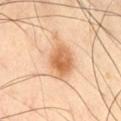A male subject aged approximately 55. A roughly 15 mm field-of-view crop from a total-body skin photograph. Located on the left thigh.